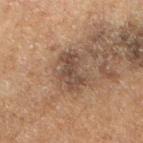The lesion was photographed on a routine skin check and not biopsied; there is no pathology result.
A female subject, approximately 50 years of age.
A region of skin cropped from a whole-body photographic capture, roughly 15 mm wide.
The recorded lesion diameter is about 4 mm.
The lesion is located on the arm.